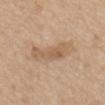Q: Is there a histopathology result?
A: imaged on a skin check; not biopsied
Q: Lesion location?
A: the mid back
Q: How was the tile lit?
A: white-light illumination
Q: What kind of image is this?
A: ~15 mm tile from a whole-body skin photo
Q: Lesion size?
A: ≈6 mm
Q: Automated lesion metrics?
A: a lesion area of about 13 mm², an outline eccentricity of about 0.9 (0 = round, 1 = elongated), and two-axis asymmetry of about 0.3; a classifier nevus-likeness of about 0/100 and a detector confidence of about 100 out of 100 that the crop contains a lesion
Q: What are the patient's age and sex?
A: female, aged 68 to 72